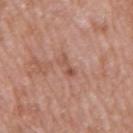Q: What kind of image is this?
A: ~15 mm crop, total-body skin-cancer survey
Q: What did automated image analysis measure?
A: border irregularity of about 5 on a 0–10 scale, a color-variation rating of about 0.5/10, and radial color variation of about 0; a lesion-detection confidence of about 100/100
Q: How large is the lesion?
A: about 3.5 mm
Q: Who is the patient?
A: male, aged 58 to 62
Q: Where on the body is the lesion?
A: the right upper arm